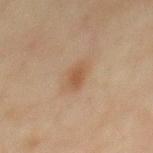Impression: The lesion was photographed on a routine skin check and not biopsied; there is no pathology result.